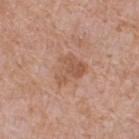Q: Was a biopsy performed?
A: total-body-photography surveillance lesion; no biopsy
Q: How was the tile lit?
A: white-light illumination
Q: What did automated image analysis measure?
A: a footprint of about 8.5 mm², an eccentricity of roughly 0.55, and a shape-asymmetry score of about 0.4 (0 = symmetric); a lesion color around L≈55 a*≈21 b*≈31 in CIELAB and roughly 8 lightness units darker than nearby skin; a border-irregularity index near 4/10, a within-lesion color-variation index near 4/10, and a peripheral color-asymmetry measure near 1.5; a classifier nevus-likeness of about 5/100 and a lesion-detection confidence of about 100/100
Q: What is the imaging modality?
A: ~15 mm tile from a whole-body skin photo
Q: Lesion location?
A: the back
Q: Patient demographics?
A: male, aged approximately 65
Q: What is the lesion's diameter?
A: ≈4 mm